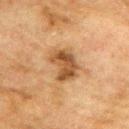This lesion was catalogued during total-body skin photography and was not selected for biopsy.
The lesion is located on the back.
The recorded lesion diameter is about 4.5 mm.
This image is a 15 mm lesion crop taken from a total-body photograph.
A male patient, in their mid- to late 70s.